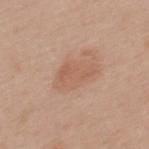Assessment:
The lesion was tiled from a total-body skin photograph and was not biopsied.
Context:
On the upper back. The tile uses white-light illumination. A close-up tile cropped from a whole-body skin photograph, about 15 mm across. The subject is a female about 40 years old.